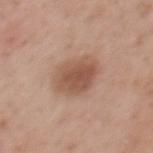  biopsy_status: not biopsied; imaged during a skin examination